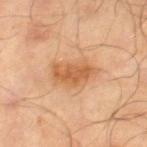This image is a 15 mm lesion crop taken from a total-body photograph. The lesion is on the right lower leg. The tile uses cross-polarized illumination. About 5 mm across. The total-body-photography lesion software estimated a footprint of about 11 mm², an outline eccentricity of about 0.8 (0 = round, 1 = elongated), and two-axis asymmetry of about 0.25. The analysis additionally found a color-variation rating of about 3/10 and peripheral color asymmetry of about 1. The analysis additionally found a lesion-detection confidence of about 100/100. A male subject in their mid- to late 60s.